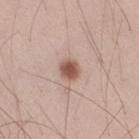follow-up=imaged on a skin check; not biopsied
automated metrics=a shape eccentricity near 0.45 and a symmetry-axis asymmetry near 0.2; border irregularity of about 1.5 on a 0–10 scale, a color-variation rating of about 2.5/10, and peripheral color asymmetry of about 0.5; an automated nevus-likeness rating near 95 out of 100 and a lesion-detection confidence of about 100/100
lighting=white-light
subject=male, aged around 30
acquisition=total-body-photography crop, ~15 mm field of view
site=the right thigh
diameter=about 2.5 mm